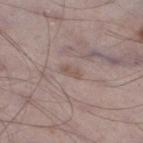  biopsy_status: not biopsied; imaged during a skin examination
  site: left lower leg
  lesion_size:
    long_diameter_mm_approx: 2.5
  patient:
    sex: male
    age_approx: 70
  lighting: white-light
  image:
    source: total-body photography crop
    field_of_view_mm: 15